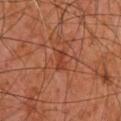Case summary:
– notes — catalogued during a skin exam; not biopsied
– size — ≈2.5 mm
– imaging modality — ~15 mm crop, total-body skin-cancer survey
– patient — male, approximately 60 years of age
– anatomic site — the back